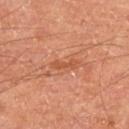Q: Was this lesion biopsied?
A: total-body-photography surveillance lesion; no biopsy
Q: How was this image acquired?
A: 15 mm crop, total-body photography
Q: What lighting was used for the tile?
A: cross-polarized
Q: Lesion size?
A: about 3 mm
Q: Patient demographics?
A: male, aged 63 to 67
Q: Lesion location?
A: the upper back